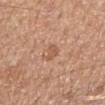– notes · no biopsy performed (imaged during a skin exam)
– illumination · white-light
– size · about 3 mm
– image-analysis metrics · a footprint of about 3 mm², an eccentricity of roughly 0.9, and a shape-asymmetry score of about 0.3 (0 = symmetric)
– anatomic site · the arm
– acquisition · total-body-photography crop, ~15 mm field of view
– subject · female, roughly 50 years of age